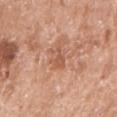Part of a total-body skin-imaging series; this lesion was reviewed on a skin check and was not flagged for biopsy.
Imaged with white-light lighting.
About 3 mm across.
On the right upper arm.
The subject is a female about 75 years old.
A 15 mm close-up extracted from a 3D total-body photography capture.